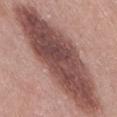Impression:
This lesion was catalogued during total-body skin photography and was not selected for biopsy.
Background:
Longest diameter approximately 14.5 mm. A 15 mm crop from a total-body photograph taken for skin-cancer surveillance. Imaged with white-light lighting. The subject is a female approximately 40 years of age. The lesion is located on the leg.